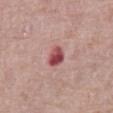Notes:
– body site: the abdomen
– automated lesion analysis: a footprint of about 4.5 mm² and a symmetry-axis asymmetry near 0.25; a border-irregularity rating of about 2/10, a color-variation rating of about 6.5/10, and peripheral color asymmetry of about 2.5
– size: ≈3 mm
– image source: total-body-photography crop, ~15 mm field of view
– subject: male, in their 70s
– tile lighting: white-light